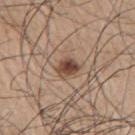biopsy_status: not biopsied; imaged during a skin examination
site: right upper arm
image:
  source: total-body photography crop
  field_of_view_mm: 15
lesion_size:
  long_diameter_mm_approx: 2.5
patient:
  sex: male
  age_approx: 75